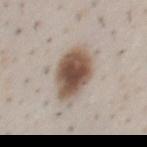Q: Was a biopsy performed?
A: catalogued during a skin exam; not biopsied
Q: What is the lesion's diameter?
A: ≈4.5 mm
Q: What did automated image analysis measure?
A: a lesion area of about 17 mm², an outline eccentricity of about 0.5 (0 = round, 1 = elongated), and a symmetry-axis asymmetry near 0.15; a nevus-likeness score of about 100/100 and a detector confidence of about 100 out of 100 that the crop contains a lesion
Q: What is the anatomic site?
A: the front of the torso
Q: Who is the patient?
A: male, aged 48 to 52
Q: What is the imaging modality?
A: total-body-photography crop, ~15 mm field of view